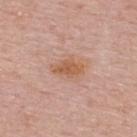This lesion was catalogued during total-body skin photography and was not selected for biopsy. A female patient about 50 years old. Automated image analysis of the tile measured an area of roughly 6.5 mm², a shape eccentricity near 0.85, and two-axis asymmetry of about 0.25. And it measured a color-variation rating of about 2.5/10 and radial color variation of about 1. And it measured a detector confidence of about 100 out of 100 that the crop contains a lesion. This is a white-light tile. Located on the upper back. The lesion's longest dimension is about 4 mm. A 15 mm close-up extracted from a 3D total-body photography capture.